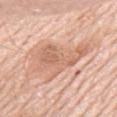Part of a total-body skin-imaging series; this lesion was reviewed on a skin check and was not flagged for biopsy.
A male subject, aged 78–82.
Located on the chest.
A lesion tile, about 15 mm wide, cut from a 3D total-body photograph.